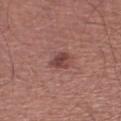workup: catalogued during a skin exam; not biopsied | automated metrics: an average lesion color of about L≈43 a*≈24 b*≈22 (CIELAB), roughly 10 lightness units darker than nearby skin, and a lesion-to-skin contrast of about 8 (normalized; higher = more distinct); border irregularity of about 2.5 on a 0–10 scale and a color-variation rating of about 3.5/10; a classifier nevus-likeness of about 70/100 and a lesion-detection confidence of about 100/100 | patient: male, about 65 years old | image: 15 mm crop, total-body photography | body site: the leg.